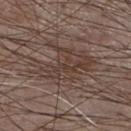This lesion was catalogued during total-body skin photography and was not selected for biopsy. Longest diameter approximately 7 mm. A male patient, in their mid- to late 70s. The lesion is on the chest. This image is a 15 mm lesion crop taken from a total-body photograph. This is a white-light tile.